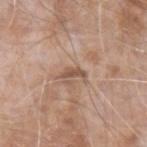Imaged during a routine full-body skin examination; the lesion was not biopsied and no histopathology is available.
The subject is a male in their mid- to late 70s.
Automated image analysis of the tile measured a lesion area of about 2.5 mm², a shape eccentricity near 0.9, and a symmetry-axis asymmetry near 0.45. The software also gave border irregularity of about 4.5 on a 0–10 scale, a within-lesion color-variation index near 0/10, and radial color variation of about 0.
Measured at roughly 3 mm in maximum diameter.
This is a white-light tile.
The lesion is located on the left forearm.
A lesion tile, about 15 mm wide, cut from a 3D total-body photograph.